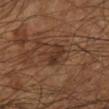Assessment:
The lesion was photographed on a routine skin check and not biopsied; there is no pathology result.
Context:
A close-up tile cropped from a whole-body skin photograph, about 15 mm across. A male patient aged approximately 55. The lesion is located on the left lower leg.